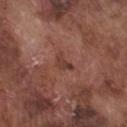follow-up: total-body-photography surveillance lesion; no biopsy
acquisition: 15 mm crop, total-body photography
anatomic site: the chest
lighting: white-light
subject: male, in their mid- to late 70s
diameter: ≈2.5 mm